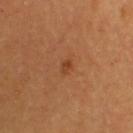<tbp_lesion>
  <biopsy_status>not biopsied; imaged during a skin examination</biopsy_status>
</tbp_lesion>